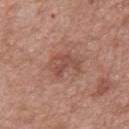Imaged during a routine full-body skin examination; the lesion was not biopsied and no histopathology is available. The lesion-visualizer software estimated a nevus-likeness score of about 0/100 and a detector confidence of about 100 out of 100 that the crop contains a lesion. Longest diameter approximately 3.5 mm. This is a white-light tile. Located on the back. The subject is a male approximately 50 years of age. Cropped from a whole-body photographic skin survey; the tile spans about 15 mm.